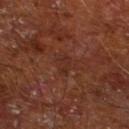Recorded during total-body skin imaging; not selected for excision or biopsy.
A male subject about 65 years old.
From the left lower leg.
Approximately 2.5 mm at its widest.
A close-up tile cropped from a whole-body skin photograph, about 15 mm across.